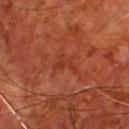* biopsy status — no biopsy performed (imaged during a skin exam)
* subject — male, aged 58 to 62
* acquisition — 15 mm crop, total-body photography
* site — the chest